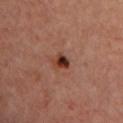Assessment:
Part of a total-body skin-imaging series; this lesion was reviewed on a skin check and was not flagged for biopsy.
Acquisition and patient details:
The lesion's longest dimension is about 2 mm. The subject is a female aged 43 to 47. On the chest. A region of skin cropped from a whole-body photographic capture, roughly 15 mm wide. Imaged with cross-polarized lighting.